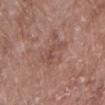An algorithmic analysis of the crop reported an average lesion color of about L≈48 a*≈20 b*≈25 (CIELAB), roughly 7 lightness units darker than nearby skin, and a normalized lesion–skin contrast near 5. The analysis additionally found a nevus-likeness score of about 0/100 and lesion-presence confidence of about 100/100. The lesion's longest dimension is about 3 mm. Captured under white-light illumination. A female patient roughly 70 years of age. A 15 mm crop from a total-body photograph taken for skin-cancer surveillance. Located on the front of the torso.